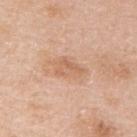Impression: Captured during whole-body skin photography for melanoma surveillance; the lesion was not biopsied. Clinical summary: Approximately 4 mm at its widest. This is a white-light tile. The lesion-visualizer software estimated a footprint of about 7 mm², an eccentricity of roughly 0.8, and two-axis asymmetry of about 0.25. And it measured a lesion color around L≈63 a*≈21 b*≈33 in CIELAB, about 8 CIELAB-L* units darker than the surrounding skin, and a normalized lesion–skin contrast near 5.5. It also reported peripheral color asymmetry of about 1. It also reported a classifier nevus-likeness of about 0/100 and a lesion-detection confidence of about 100/100. Cropped from a whole-body photographic skin survey; the tile spans about 15 mm. Located on the arm. A female subject, in their mid- to late 40s.